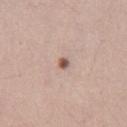Q: Was this lesion biopsied?
A: total-body-photography surveillance lesion; no biopsy
Q: Lesion size?
A: about 1.5 mm
Q: What are the patient's age and sex?
A: female, aged 18 to 22
Q: What is the imaging modality?
A: total-body-photography crop, ~15 mm field of view
Q: What is the anatomic site?
A: the right thigh